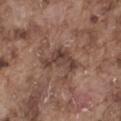{"biopsy_status": "not biopsied; imaged during a skin examination", "lighting": "white-light", "patient": {"sex": "male", "age_approx": 75}, "lesion_size": {"long_diameter_mm_approx": 5.0}, "image": {"source": "total-body photography crop", "field_of_view_mm": 15}, "site": "abdomen"}